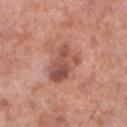Captured during whole-body skin photography for melanoma surveillance; the lesion was not biopsied.
A male subject aged 53–57.
Located on the left lower leg.
A 15 mm close-up extracted from a 3D total-body photography capture.
The lesion's longest dimension is about 4.5 mm.
This is a white-light tile.
Automated tile analysis of the lesion measured a lesion color around L≈52 a*≈25 b*≈28 in CIELAB, a lesion–skin lightness drop of about 11, and a normalized lesion–skin contrast near 7.5.